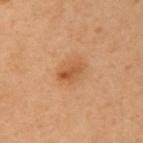Clinical impression:
This lesion was catalogued during total-body skin photography and was not selected for biopsy.
Clinical summary:
A female patient aged 58–62. Cropped from a total-body skin-imaging series; the visible field is about 15 mm. Located on the chest.